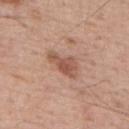Notes:
• biopsy status · no biopsy performed (imaged during a skin exam)
• image-analysis metrics · a lesion area of about 6.5 mm², a shape eccentricity near 0.9, and a shape-asymmetry score of about 0.4 (0 = symmetric); roughly 10 lightness units darker than nearby skin and a normalized lesion–skin contrast near 7; a border-irregularity index near 4.5/10, a within-lesion color-variation index near 3/10, and radial color variation of about 1; a lesion-detection confidence of about 100/100
• location · the upper back
• subject · male, roughly 55 years of age
• lighting · white-light
• image · ~15 mm tile from a whole-body skin photo
• lesion diameter · ~4.5 mm (longest diameter)